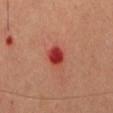{
  "biopsy_status": "not biopsied; imaged during a skin examination",
  "automated_metrics": {
    "lesion_detection_confidence_0_100": 100
  },
  "patient": {
    "sex": "male",
    "age_approx": 60
  },
  "site": "abdomen",
  "image": {
    "source": "total-body photography crop",
    "field_of_view_mm": 15
  }
}